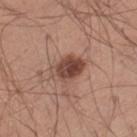Impression: Imaged during a routine full-body skin examination; the lesion was not biopsied and no histopathology is available. Acquisition and patient details: The lesion is located on the left thigh. The patient is a male in their 40s. The lesion-visualizer software estimated a lesion–skin lightness drop of about 14 and a lesion-to-skin contrast of about 10 (normalized; higher = more distinct). It also reported a nevus-likeness score of about 85/100 and a lesion-detection confidence of about 100/100. A 15 mm crop from a total-body photograph taken for skin-cancer surveillance. The tile uses white-light illumination.